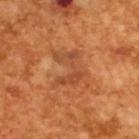• biopsy status · imaged on a skin check; not biopsied
• acquisition · ~15 mm crop, total-body skin-cancer survey
• subject · male, aged 63 to 67
• image-analysis metrics · an area of roughly 7.5 mm²; about 7 CIELAB-L* units darker than the surrounding skin and a normalized border contrast of about 5.5; border irregularity of about 8.5 on a 0–10 scale, a color-variation rating of about 3.5/10, and radial color variation of about 1; a classifier nevus-likeness of about 0/100
• lighting · cross-polarized illumination
• lesion size · ≈4 mm